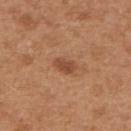  biopsy_status: not biopsied; imaged during a skin examination
  lesion_size:
    long_diameter_mm_approx: 3.0
  patient:
    sex: female
    age_approx: 40
  site: upper back
  image:
    source: total-body photography crop
    field_of_view_mm: 15
  automated_metrics:
    area_mm2_approx: 3.5
    eccentricity: 0.8